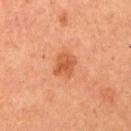Q: Is there a histopathology result?
A: imaged on a skin check; not biopsied
Q: Lesion location?
A: the right upper arm
Q: Patient demographics?
A: female, aged 58 to 62
Q: What kind of image is this?
A: 15 mm crop, total-body photography